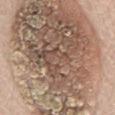biopsy status: no biopsy performed (imaged during a skin exam)
imaging modality: ~15 mm crop, total-body skin-cancer survey
site: the front of the torso
lesion size: ~20.5 mm (longest diameter)
subject: male, approximately 75 years of age
lighting: white-light